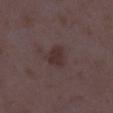Q: What is the imaging modality?
A: total-body-photography crop, ~15 mm field of view
Q: What is the lesion's diameter?
A: ~2.5 mm (longest diameter)
Q: Who is the patient?
A: female, aged around 35
Q: Lesion location?
A: the right lower leg
Q: What lighting was used for the tile?
A: white-light illumination
Q: What did automated image analysis measure?
A: an area of roughly 5 mm², an outline eccentricity of about 0.45 (0 = round, 1 = elongated), and a symmetry-axis asymmetry near 0.25; a lesion color around L≈30 a*≈16 b*≈15 in CIELAB, a lesion–skin lightness drop of about 7, and a normalized border contrast of about 7.5; a border-irregularity index near 2/10 and a within-lesion color-variation index near 1.5/10; a nevus-likeness score of about 5/100 and lesion-presence confidence of about 100/100